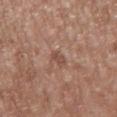Q: Was this lesion biopsied?
A: no biopsy performed (imaged during a skin exam)
Q: How was the tile lit?
A: white-light illumination
Q: How large is the lesion?
A: ≈2.5 mm
Q: What is the anatomic site?
A: the mid back
Q: What did automated image analysis measure?
A: a footprint of about 2.5 mm², an eccentricity of roughly 0.9, and a shape-asymmetry score of about 0.3 (0 = symmetric); a border-irregularity rating of about 3/10 and peripheral color asymmetry of about 0; lesion-presence confidence of about 95/100
Q: Who is the patient?
A: male, roughly 50 years of age
Q: What kind of image is this?
A: total-body-photography crop, ~15 mm field of view